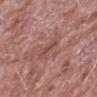Notes:
– follow-up · no biopsy performed (imaged during a skin exam)
– image source · ~15 mm tile from a whole-body skin photo
– subject · male, roughly 75 years of age
– site · the right forearm